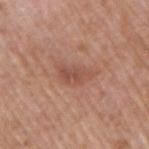workup — total-body-photography surveillance lesion; no biopsy | diameter — about 4 mm | patient — male, in their 70s | body site — the right upper arm | imaging modality — 15 mm crop, total-body photography | automated lesion analysis — a lesion area of about 6.5 mm², a shape eccentricity near 0.85, and a symmetry-axis asymmetry near 0.3; a mean CIELAB color near L≈51 a*≈24 b*≈28, about 8 CIELAB-L* units darker than the surrounding skin, and a normalized border contrast of about 6; a border-irregularity rating of about 3.5/10 and a within-lesion color-variation index near 3/10; a nevus-likeness score of about 0/100 and lesion-presence confidence of about 100/100.